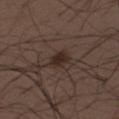follow-up — total-body-photography surveillance lesion; no biopsy | image source — total-body-photography crop, ~15 mm field of view | subject — male, approximately 30 years of age | anatomic site — the upper back.